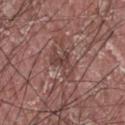The lesion was tiled from a total-body skin photograph and was not biopsied.
On the head or neck.
A male patient, about 40 years old.
The tile uses white-light illumination.
Measured at roughly 3.5 mm in maximum diameter.
Cropped from a whole-body photographic skin survey; the tile spans about 15 mm.